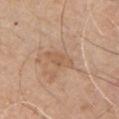Q: Was a biopsy performed?
A: no biopsy performed (imaged during a skin exam)
Q: Who is the patient?
A: male, aged 63 to 67
Q: What kind of image is this?
A: 15 mm crop, total-body photography
Q: What is the anatomic site?
A: the chest